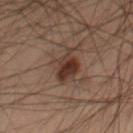Notes:
– acquisition · total-body-photography crop, ~15 mm field of view
– patient · male, in their mid-50s
– body site · the right thigh